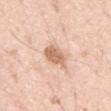<case>
<biopsy_status>not biopsied; imaged during a skin examination</biopsy_status>
<patient>
  <sex>male</sex>
  <age_approx>70</age_approx>
</patient>
<image>
  <source>total-body photography crop</source>
  <field_of_view_mm>15</field_of_view_mm>
</image>
<lesion_size>
  <long_diameter_mm_approx>3.5</long_diameter_mm_approx>
</lesion_size>
<site>abdomen</site>
</case>